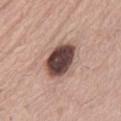Imaged during a routine full-body skin examination; the lesion was not biopsied and no histopathology is available. A male patient approximately 65 years of age. Approximately 5 mm at its widest. On the abdomen. A region of skin cropped from a whole-body photographic capture, roughly 15 mm wide. Imaged with white-light lighting.